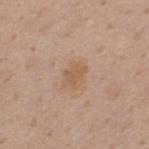Assessment: The lesion was tiled from a total-body skin photograph and was not biopsied. Background: From the upper back. A roughly 15 mm field-of-view crop from a total-body skin photograph. The total-body-photography lesion software estimated about 7 CIELAB-L* units darker than the surrounding skin and a lesion-to-skin contrast of about 5.5 (normalized; higher = more distinct). It also reported a border-irregularity index near 2.5/10, a color-variation rating of about 1.5/10, and radial color variation of about 0.5. And it measured a nevus-likeness score of about 0/100. Measured at roughly 2.5 mm in maximum diameter. A male patient in their 40s.